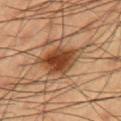  biopsy_status: not biopsied; imaged during a skin examination
  site: right upper arm
  lighting: cross-polarized
  lesion_size:
    long_diameter_mm_approx: 5.0
  patient:
    sex: male
    age_approx: 55
  automated_metrics:
    area_mm2_approx: 14.0
    shape_asymmetry: 0.3
    cielab_L: 38
    cielab_a: 20
    cielab_b: 31
    vs_skin_darker_L: 12.0
    vs_skin_contrast_norm: 10.5
    border_irregularity_0_10: 3.5
    peripheral_color_asymmetry: 1.5
    nevus_likeness_0_100: 95
    lesion_detection_confidence_0_100: 100
  image:
    source: total-body photography crop
    field_of_view_mm: 15